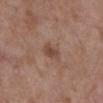workup = total-body-photography surveillance lesion; no biopsy
location = the right lower leg
image source = 15 mm crop, total-body photography
patient = female, roughly 65 years of age